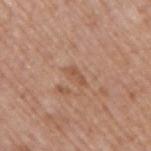Captured during whole-body skin photography for melanoma surveillance; the lesion was not biopsied. A male patient aged around 70. Measured at roughly 3 mm in maximum diameter. Automated image analysis of the tile measured a normalized lesion–skin contrast near 5.5. And it measured a classifier nevus-likeness of about 0/100 and a lesion-detection confidence of about 100/100. The lesion is located on the arm. This image is a 15 mm lesion crop taken from a total-body photograph.